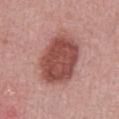This lesion was catalogued during total-body skin photography and was not selected for biopsy. A roughly 15 mm field-of-view crop from a total-body skin photograph. Longest diameter approximately 7 mm. From the abdomen. The subject is a male approximately 50 years of age.